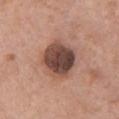Impression: Part of a total-body skin-imaging series; this lesion was reviewed on a skin check and was not flagged for biopsy. Image and clinical context: A region of skin cropped from a whole-body photographic capture, roughly 15 mm wide. The tile uses white-light illumination. On the chest. A female subject aged approximately 40. Measured at roughly 4.5 mm in maximum diameter. An algorithmic analysis of the crop reported a lesion area of about 18 mm² and a symmetry-axis asymmetry near 0.15. And it measured an average lesion color of about L≈46 a*≈20 b*≈24 (CIELAB), about 17 CIELAB-L* units darker than the surrounding skin, and a normalized border contrast of about 12. The analysis additionally found a border-irregularity index near 1.5/10, internal color variation of about 6 on a 0–10 scale, and peripheral color asymmetry of about 2. And it measured a classifier nevus-likeness of about 5/100 and a lesion-detection confidence of about 100/100.